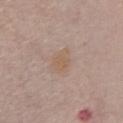Findings:
– follow-up: total-body-photography surveillance lesion; no biopsy
– subject: female, roughly 65 years of age
– anatomic site: the chest
– automated lesion analysis: roughly 5 lightness units darker than nearby skin and a lesion-to-skin contrast of about 5.5 (normalized; higher = more distinct); a nevus-likeness score of about 5/100 and a lesion-detection confidence of about 100/100
– lesion size: ~3 mm (longest diameter)
– image source: ~15 mm tile from a whole-body skin photo